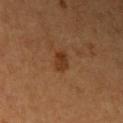workup — catalogued during a skin exam; not biopsied
location — the left upper arm
tile lighting — cross-polarized
size — ≈2.5 mm
patient — female, in their 60s
image — total-body-photography crop, ~15 mm field of view